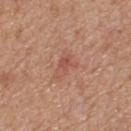Case summary:
– biopsy status — total-body-photography surveillance lesion; no biopsy
– image source — total-body-photography crop, ~15 mm field of view
– diameter — ≈3 mm
– site — the upper back
– subject — male, aged approximately 55
– lighting — white-light
– automated metrics — a lesion area of about 4 mm², an outline eccentricity of about 0.8 (0 = round, 1 = elongated), and a shape-asymmetry score of about 0.35 (0 = symmetric); a mean CIELAB color near L≈52 a*≈26 b*≈28, about 7 CIELAB-L* units darker than the surrounding skin, and a lesion-to-skin contrast of about 5 (normalized; higher = more distinct); a border-irregularity index near 4/10 and a within-lesion color-variation index near 4/10